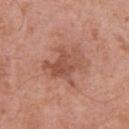biopsy status = no biopsy performed (imaged during a skin exam)
illumination = white-light illumination
subject = male, in their 70s
lesion size = ~4.5 mm (longest diameter)
site = the chest
image = total-body-photography crop, ~15 mm field of view